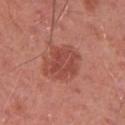Assessment:
The lesion was photographed on a routine skin check and not biopsied; there is no pathology result.